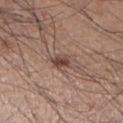Part of a total-body skin-imaging series; this lesion was reviewed on a skin check and was not flagged for biopsy. A 15 mm close-up tile from a total-body photography series done for melanoma screening. The lesion-visualizer software estimated an eccentricity of roughly 0.7 and two-axis asymmetry of about 0.25. The software also gave a lesion–skin lightness drop of about 12 and a lesion-to-skin contrast of about 9 (normalized; higher = more distinct). And it measured border irregularity of about 3 on a 0–10 scale, a color-variation rating of about 2.5/10, and radial color variation of about 1. And it measured a lesion-detection confidence of about 100/100. Measured at roughly 2.5 mm in maximum diameter. From the leg. This is a white-light tile. A male patient aged 33 to 37.